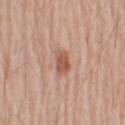notes: catalogued during a skin exam; not biopsied | site: the right upper arm | lesion size: about 3 mm | tile lighting: white-light illumination | subject: male, roughly 55 years of age | imaging modality: ~15 mm tile from a whole-body skin photo.